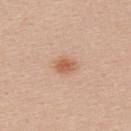This lesion was catalogued during total-body skin photography and was not selected for biopsy. The lesion is on the back. A 15 mm close-up extracted from a 3D total-body photography capture. A female patient in their 20s.